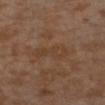Context: An algorithmic analysis of the crop reported a footprint of about 7 mm² and an eccentricity of roughly 0.95. It also reported an average lesion color of about L≈37 a*≈17 b*≈28 (CIELAB) and a lesion-to-skin contrast of about 5 (normalized; higher = more distinct). The software also gave a detector confidence of about 100 out of 100 that the crop contains a lesion. Measured at roughly 4.5 mm in maximum diameter. The patient is a male aged around 30. On the left lower leg. A 15 mm close-up tile from a total-body photography series done for melanoma screening. This is a cross-polarized tile.